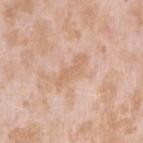workup = imaged on a skin check; not biopsied
patient = female, aged 23–27
acquisition = 15 mm crop, total-body photography
body site = the right upper arm